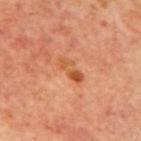{
  "biopsy_status": "not biopsied; imaged during a skin examination",
  "patient": {
    "sex": "male",
    "age_approx": 70
  },
  "site": "mid back",
  "automated_metrics": {
    "area_mm2_approx": 5.0,
    "eccentricity": 0.9,
    "cielab_L": 53,
    "cielab_a": 28,
    "cielab_b": 40,
    "vs_skin_darker_L": 9.0,
    "vs_skin_contrast_norm": 7.0
  },
  "image": {
    "source": "total-body photography crop",
    "field_of_view_mm": 15
  },
  "lighting": "cross-polarized",
  "lesion_size": {
    "long_diameter_mm_approx": 3.5
  }
}